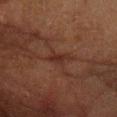The lesion was tiled from a total-body skin photograph and was not biopsied.
On the right forearm.
The total-body-photography lesion software estimated a lesion color around L≈23 a*≈18 b*≈21 in CIELAB and a normalized border contrast of about 6.5. The software also gave border irregularity of about 3 on a 0–10 scale, internal color variation of about 0.5 on a 0–10 scale, and a peripheral color-asymmetry measure near 0.
Cropped from a total-body skin-imaging series; the visible field is about 15 mm.
The lesion's longest dimension is about 2.5 mm.
The tile uses cross-polarized illumination.
A male patient in their mid- to late 60s.